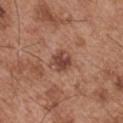follow-up: catalogued during a skin exam; not biopsied | lighting: white-light illumination | location: the arm | image-analysis metrics: a lesion area of about 6.5 mm², a shape eccentricity near 0.5, and two-axis asymmetry of about 0.25; a lesion–skin lightness drop of about 11 and a lesion-to-skin contrast of about 8 (normalized; higher = more distinct); a nevus-likeness score of about 15/100 | subject: male, aged 53–57 | acquisition: ~15 mm tile from a whole-body skin photo.